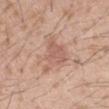Assessment:
Recorded during total-body skin imaging; not selected for excision or biopsy.
Context:
A male patient, aged around 25. A roughly 15 mm field-of-view crop from a total-body skin photograph. The lesion-visualizer software estimated an automated nevus-likeness rating near 0 out of 100 and lesion-presence confidence of about 100/100. Captured under white-light illumination. The lesion is located on the left forearm. The recorded lesion diameter is about 5.5 mm.